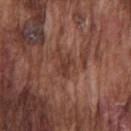Imaged during a routine full-body skin examination; the lesion was not biopsied and no histopathology is available. A region of skin cropped from a whole-body photographic capture, roughly 15 mm wide. The lesion is located on the chest. A male patient about 75 years old.